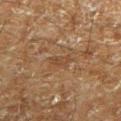<tbp_lesion>
<automated_metrics>
  <cielab_L>34</cielab_L>
  <cielab_a>15</cielab_a>
  <cielab_b>25</cielab_b>
  <vs_skin_darker_L>5.0</vs_skin_darker_L>
  <vs_skin_contrast_norm>5.5</vs_skin_contrast_norm>
  <border_irregularity_0_10>3.5</border_irregularity_0_10>
  <color_variation_0_10>0.0</color_variation_0_10>
</automated_metrics>
<lighting>cross-polarized</lighting>
<lesion_size>
  <long_diameter_mm_approx>2.5</long_diameter_mm_approx>
</lesion_size>
<image>
  <source>total-body photography crop</source>
  <field_of_view_mm>15</field_of_view_mm>
</image>
<site>leg</site>
<patient>
  <sex>male</sex>
  <age_approx>60</age_approx>
</patient>
</tbp_lesion>